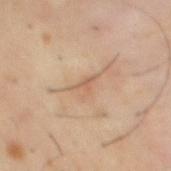biopsy status: catalogued during a skin exam; not biopsied
acquisition: ~15 mm tile from a whole-body skin photo
TBP lesion metrics: a border-irregularity index near 6.5/10, internal color variation of about 1.5 on a 0–10 scale, and a peripheral color-asymmetry measure near 0.5
lighting: cross-polarized illumination
patient: male, aged 38–42
site: the mid back
diameter: ~3.5 mm (longest diameter)